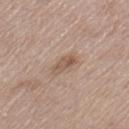Imaged with white-light lighting. The subject is a female in their mid- to late 60s. Located on the left thigh. Automated image analysis of the tile measured an area of roughly 4 mm², an outline eccentricity of about 0.85 (0 = round, 1 = elongated), and two-axis asymmetry of about 0.25. The analysis additionally found a mean CIELAB color near L≈55 a*≈17 b*≈27. The analysis additionally found a border-irregularity rating of about 2.5/10, a within-lesion color-variation index near 4/10, and peripheral color asymmetry of about 1.5. This image is a 15 mm lesion crop taken from a total-body photograph. The recorded lesion diameter is about 3 mm.